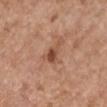The lesion was photographed on a routine skin check and not biopsied; there is no pathology result. A roughly 15 mm field-of-view crop from a total-body skin photograph. Measured at roughly 4 mm in maximum diameter. Located on the right upper arm. The total-body-photography lesion software estimated a lesion color around L≈50 a*≈23 b*≈31 in CIELAB and about 10 CIELAB-L* units darker than the surrounding skin. The analysis additionally found a border-irregularity rating of about 5.5/10 and radial color variation of about 1. Captured under white-light illumination. The patient is a female approximately 65 years of age.